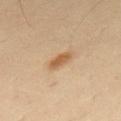Impression:
Part of a total-body skin-imaging series; this lesion was reviewed on a skin check and was not flagged for biopsy.
Clinical summary:
On the upper back. A 15 mm crop from a total-body photograph taken for skin-cancer surveillance. The patient is a male aged around 50.